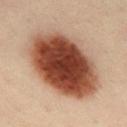lesion_size:
  long_diameter_mm_approx: 10.0
site: mid back
automated_metrics:
  area_mm2_approx: 50.0
  shape_asymmetry: 0.05
  border_irregularity_0_10: 1.0
  nevus_likeness_0_100: 100
  lesion_detection_confidence_0_100: 100
image:
  source: total-body photography crop
  field_of_view_mm: 15
lighting: cross-polarized
patient:
  sex: male
  age_approx: 50